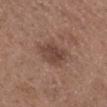| key | value |
|---|---|
| biopsy status | total-body-photography surveillance lesion; no biopsy |
| image | ~15 mm tile from a whole-body skin photo |
| lesion size | ≈4.5 mm |
| subject | male, aged around 35 |
| lighting | white-light illumination |
| location | the head or neck |